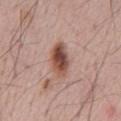Findings:
• workup: no biopsy performed (imaged during a skin exam)
• patient: male, in their mid- to late 60s
• automated metrics: an area of roughly 9 mm², an outline eccentricity of about 0.85 (0 = round, 1 = elongated), and a shape-asymmetry score of about 0.15 (0 = symmetric); an average lesion color of about L≈50 a*≈21 b*≈26 (CIELAB), a lesion–skin lightness drop of about 15, and a lesion-to-skin contrast of about 10.5 (normalized; higher = more distinct)
• imaging modality: ~15 mm crop, total-body skin-cancer survey
• lighting: white-light illumination
• lesion size: ~4.5 mm (longest diameter)
• body site: the mid back